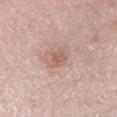Findings:
• workup: total-body-photography surveillance lesion; no biopsy